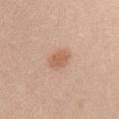Impression:
Captured during whole-body skin photography for melanoma surveillance; the lesion was not biopsied.
Clinical summary:
The subject is a female aged around 25. A 15 mm close-up tile from a total-body photography series done for melanoma screening. The lesion is on the right upper arm. This is a white-light tile. The recorded lesion diameter is about 3 mm.